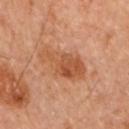Findings:
- notes · catalogued during a skin exam; not biopsied
- image source · 15 mm crop, total-body photography
- anatomic site · the arm
- patient · female, roughly 60 years of age
- tile lighting · cross-polarized illumination
- lesion size · ≈5 mm